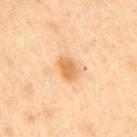Part of a total-body skin-imaging series; this lesion was reviewed on a skin check and was not flagged for biopsy. From the arm. A region of skin cropped from a whole-body photographic capture, roughly 15 mm wide. The subject is a male approximately 40 years of age.